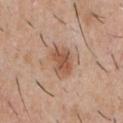No biopsy was performed on this lesion — it was imaged during a full skin examination and was not determined to be concerning. A roughly 15 mm field-of-view crop from a total-body skin photograph. A male subject, in their 30s. Located on the chest.